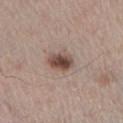  biopsy_status: not biopsied; imaged during a skin examination
  automated_metrics:
    nevus_likeness_0_100: 95
    lesion_detection_confidence_0_100: 100
  lighting: white-light
  patient:
    sex: male
    age_approx: 60
  site: right lower leg
  image:
    source: total-body photography crop
    field_of_view_mm: 15